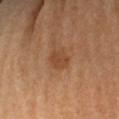Impression:
The lesion was photographed on a routine skin check and not biopsied; there is no pathology result.
Background:
About 3 mm across. Automated tile analysis of the lesion measured internal color variation of about 2 on a 0–10 scale and peripheral color asymmetry of about 0.5. It also reported an automated nevus-likeness rating near 10 out of 100 and a lesion-detection confidence of about 100/100. Located on the left arm. The tile uses cross-polarized illumination. A 15 mm crop from a total-body photograph taken for skin-cancer surveillance. A female subject, roughly 65 years of age.